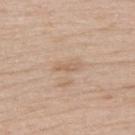lesion size: ≈2.5 mm
location: the upper back
image-analysis metrics: a mean CIELAB color near L≈61 a*≈17 b*≈31, a lesion–skin lightness drop of about 7, and a normalized lesion–skin contrast near 5; a border-irregularity rating of about 3.5/10, a within-lesion color-variation index near 0/10, and a peripheral color-asymmetry measure near 0; a classifier nevus-likeness of about 0/100
subject: female, in their 50s
acquisition: total-body-photography crop, ~15 mm field of view